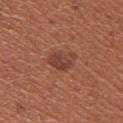No biopsy was performed on this lesion — it was imaged during a full skin examination and was not determined to be concerning. A roughly 15 mm field-of-view crop from a total-body skin photograph. Located on the left upper arm. Measured at roughly 3.5 mm in maximum diameter. This is a white-light tile. A female patient aged 33–37. An algorithmic analysis of the crop reported a lesion color around L≈41 a*≈25 b*≈28 in CIELAB and a normalized border contrast of about 7.